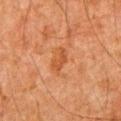diameter=≈3 mm | image source=total-body-photography crop, ~15 mm field of view | lighting=cross-polarized | TBP lesion metrics=an area of roughly 3.5 mm², an eccentricity of roughly 0.85, and a symmetry-axis asymmetry near 0.45; a border-irregularity rating of about 4.5/10, a color-variation rating of about 0.5/10, and peripheral color asymmetry of about 0 | patient=male, aged approximately 80 | body site=the abdomen.